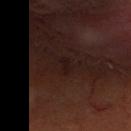Clinical impression:
This lesion was catalogued during total-body skin photography and was not selected for biopsy.
Context:
A male subject, in their mid- to late 60s. From the head or neck. A 15 mm close-up extracted from a 3D total-body photography capture. This is a cross-polarized tile.